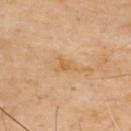biopsy_status: not biopsied; imaged during a skin examination
automated_metrics:
  area_mm2_approx: 3.5
  eccentricity: 0.8
  shape_asymmetry: 0.45
  cielab_L: 62
  cielab_a: 19
  cielab_b: 41
  vs_skin_contrast_norm: 6.0
image:
  source: total-body photography crop
  field_of_view_mm: 15
site: upper back
lighting: cross-polarized
patient:
  sex: male
  age_approx: 65